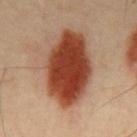Captured during whole-body skin photography for melanoma surveillance; the lesion was not biopsied. The lesion is on the mid back. A roughly 15 mm field-of-view crop from a total-body skin photograph. The lesion-visualizer software estimated an area of roughly 34 mm² and an outline eccentricity of about 0.85 (0 = round, 1 = elongated). The lesion's longest dimension is about 9 mm. The tile uses cross-polarized illumination. A male patient in their mid- to late 40s.